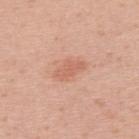Part of a total-body skin-imaging series; this lesion was reviewed on a skin check and was not flagged for biopsy.
A female subject, about 30 years old.
Imaged with white-light lighting.
Cropped from a whole-body photographic skin survey; the tile spans about 15 mm.
The lesion is on the upper back.
The total-body-photography lesion software estimated a lesion color around L≈62 a*≈25 b*≈31 in CIELAB, a lesion–skin lightness drop of about 8, and a normalized border contrast of about 5.5. The analysis additionally found a classifier nevus-likeness of about 30/100.